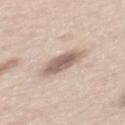– biopsy status · no biopsy performed (imaged during a skin exam)
– automated lesion analysis · a footprint of about 9 mm² and a shape eccentricity near 0.85; an average lesion color of about L≈61 a*≈15 b*≈24 (CIELAB), about 14 CIELAB-L* units darker than the surrounding skin, and a normalized lesion–skin contrast near 9; a border-irregularity index near 2.5/10 and peripheral color asymmetry of about 1; lesion-presence confidence of about 95/100
– size · ≈4.5 mm
– patient · male, aged 43 to 47
– location · the mid back
– image source · 15 mm crop, total-body photography